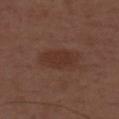Findings:
• notes — no biopsy performed (imaged during a skin exam)
• diameter — ~5 mm (longest diameter)
• TBP lesion metrics — a lesion area of about 9.5 mm², an outline eccentricity of about 0.9 (0 = round, 1 = elongated), and two-axis asymmetry of about 0.15; internal color variation of about 1.5 on a 0–10 scale and a peripheral color-asymmetry measure near 0.5; a classifier nevus-likeness of about 65/100 and a detector confidence of about 100 out of 100 that the crop contains a lesion
• imaging modality — ~15 mm tile from a whole-body skin photo
• lighting — white-light
• patient — male, approximately 50 years of age